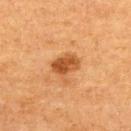Q: Who is the patient?
A: female, aged 53 to 57
Q: What lighting was used for the tile?
A: cross-polarized
Q: Automated lesion metrics?
A: a shape eccentricity near 0.7 and a shape-asymmetry score of about 0.25 (0 = symmetric)
Q: What is the imaging modality?
A: total-body-photography crop, ~15 mm field of view
Q: Lesion location?
A: the upper back